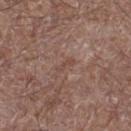The lesion was tiled from a total-body skin photograph and was not biopsied.